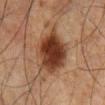Assessment:
Captured during whole-body skin photography for melanoma surveillance; the lesion was not biopsied.
Background:
The total-body-photography lesion software estimated a lesion area of about 18 mm², an eccentricity of roughly 0.5, and a symmetry-axis asymmetry near 0.2. Imaged with cross-polarized lighting. The patient is a male approximately 80 years of age. The lesion is on the front of the torso. A region of skin cropped from a whole-body photographic capture, roughly 15 mm wide. Approximately 5.5 mm at its widest.